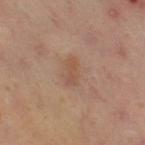subject: female, in their 40s; acquisition: ~15 mm tile from a whole-body skin photo; site: the right thigh; size: about 3 mm; lighting: cross-polarized.